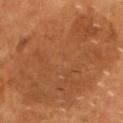notes = catalogued during a skin exam; not biopsied
patient = male, aged 58 to 62
lighting = cross-polarized
lesion size = ~15 mm (longest diameter)
image source = ~15 mm tile from a whole-body skin photo
body site = the head or neck